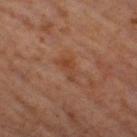{
  "biopsy_status": "not biopsied; imaged during a skin examination",
  "image": {
    "source": "total-body photography crop",
    "field_of_view_mm": 15
  },
  "site": "right thigh",
  "automated_metrics": {
    "area_mm2_approx": 3.0,
    "shape_asymmetry": 0.45
  },
  "lesion_size": {
    "long_diameter_mm_approx": 3.0
  },
  "lighting": "cross-polarized",
  "patient": {
    "sex": "male",
    "age_approx": 60
  }
}